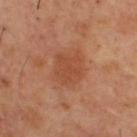Assessment: The lesion was photographed on a routine skin check and not biopsied; there is no pathology result. Image and clinical context: A male subject, aged 53–57. From the upper back. A 15 mm crop from a total-body photograph taken for skin-cancer surveillance. The tile uses cross-polarized illumination. Automated tile analysis of the lesion measured a border-irregularity index near 2.5/10 and internal color variation of about 2.5 on a 0–10 scale. It also reported an automated nevus-likeness rating near 5 out of 100 and lesion-presence confidence of about 100/100.